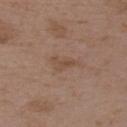Q: Was this lesion biopsied?
A: imaged on a skin check; not biopsied
Q: What is the lesion's diameter?
A: ~3.5 mm (longest diameter)
Q: How was the tile lit?
A: white-light
Q: What did automated image analysis measure?
A: a footprint of about 4 mm², an outline eccentricity of about 0.9 (0 = round, 1 = elongated), and a symmetry-axis asymmetry near 0.55; a nevus-likeness score of about 0/100 and a lesion-detection confidence of about 100/100
Q: Who is the patient?
A: female, about 35 years old
Q: What is the imaging modality?
A: ~15 mm crop, total-body skin-cancer survey
Q: What is the anatomic site?
A: the upper back